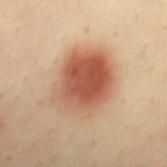No biopsy was performed on this lesion — it was imaged during a full skin examination and was not determined to be concerning. A male subject in their 50s. From the mid back. About 6.5 mm across. A lesion tile, about 15 mm wide, cut from a 3D total-body photograph.